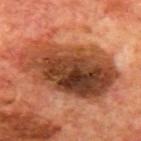The lesion was tiled from a total-body skin photograph and was not biopsied. A 15 mm close-up extracted from a 3D total-body photography capture. The lesion is located on the mid back. Longest diameter approximately 10.5 mm. A male patient aged around 70.